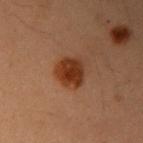This lesion was catalogued during total-body skin photography and was not selected for biopsy.
A female patient roughly 40 years of age.
The lesion is on the right upper arm.
Cropped from a whole-body photographic skin survey; the tile spans about 15 mm.